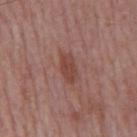follow-up: total-body-photography surveillance lesion; no biopsy
automated lesion analysis: a border-irregularity rating of about 2/10 and a color-variation rating of about 2.5/10
patient: male, roughly 75 years of age
tile lighting: white-light illumination
diameter: about 3.5 mm
image: total-body-photography crop, ~15 mm field of view
location: the mid back